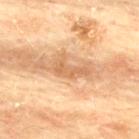– image source: 15 mm crop, total-body photography
– tile lighting: cross-polarized illumination
– automated lesion analysis: a shape eccentricity near 0.9 and a shape-asymmetry score of about 0.6 (0 = symmetric); a mean CIELAB color near L≈59 a*≈20 b*≈36, a lesion–skin lightness drop of about 10, and a lesion-to-skin contrast of about 6.5 (normalized; higher = more distinct); internal color variation of about 1.5 on a 0–10 scale and radial color variation of about 0.5; a classifier nevus-likeness of about 0/100
– patient: female, roughly 80 years of age
– lesion size: ~4 mm (longest diameter)
– anatomic site: the upper back